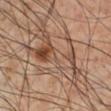Impression:
Recorded during total-body skin imaging; not selected for excision or biopsy.
Context:
The subject is a male in their mid-50s. The lesion-visualizer software estimated a within-lesion color-variation index near 10/10. And it measured a classifier nevus-likeness of about 55/100 and a detector confidence of about 65 out of 100 that the crop contains a lesion. Approximately 10 mm at its widest. A lesion tile, about 15 mm wide, cut from a 3D total-body photograph. From the right lower leg. Imaged with cross-polarized lighting.